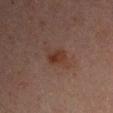Recorded during total-body skin imaging; not selected for excision or biopsy. The subject is a male roughly 30 years of age. Longest diameter approximately 2.5 mm. Cropped from a total-body skin-imaging series; the visible field is about 15 mm. On the left upper arm. The tile uses cross-polarized illumination. An algorithmic analysis of the crop reported a footprint of about 4 mm², an eccentricity of roughly 0.75, and two-axis asymmetry of about 0.25. The software also gave a lesion color around L≈27 a*≈18 b*≈21 in CIELAB, roughly 6 lightness units darker than nearby skin, and a lesion-to-skin contrast of about 7.5 (normalized; higher = more distinct).